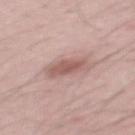No biopsy was performed on this lesion — it was imaged during a full skin examination and was not determined to be concerning.
Captured under white-light illumination.
A 15 mm close-up tile from a total-body photography series done for melanoma screening.
The lesion is on the mid back.
A male subject, aged 28–32.
Automated image analysis of the tile measured roughly 10 lightness units darker than nearby skin and a normalized lesion–skin contrast near 7.
About 3.5 mm across.